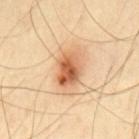Impression:
The lesion was photographed on a routine skin check and not biopsied; there is no pathology result.
Image and clinical context:
Approximately 5.5 mm at its widest. A male subject, in their mid- to late 60s. A close-up tile cropped from a whole-body skin photograph, about 15 mm across. From the mid back.